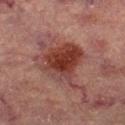Background:
About 6 mm across. A close-up tile cropped from a whole-body skin photograph, about 15 mm across. Captured under cross-polarized illumination. The lesion is located on the right lower leg. A female subject roughly 70 years of age.